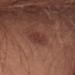follow-up: no biopsy performed (imaged during a skin exam); image source: ~15 mm tile from a whole-body skin photo; lighting: white-light illumination; patient: male, aged approximately 50; location: the front of the torso; lesion size: about 4 mm.